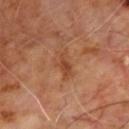Impression: This lesion was catalogued during total-body skin photography and was not selected for biopsy. Image and clinical context: A male patient aged approximately 60. This is a cross-polarized tile. Approximately 3.5 mm at its widest. The lesion is located on the upper back. A 15 mm crop from a total-body photograph taken for skin-cancer surveillance.